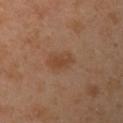biopsy_status: not biopsied; imaged during a skin examination
patient:
  sex: female
  age_approx: 40
image:
  source: total-body photography crop
  field_of_view_mm: 15
site: left upper arm
automated_metrics:
  area_mm2_approx: 4.5
  shape_asymmetry: 0.2
  cielab_L: 44
  cielab_a: 20
  cielab_b: 32
  vs_skin_darker_L: 7.0
  vs_skin_contrast_norm: 6.5
  color_variation_0_10: 2.5
  peripheral_color_asymmetry: 1.0
  nevus_likeness_0_100: 25